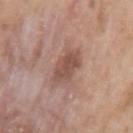{
  "biopsy_status": "not biopsied; imaged during a skin examination",
  "automated_metrics": {
    "area_mm2_approx": 8.0,
    "eccentricity": 0.75,
    "shape_asymmetry": 0.2,
    "border_irregularity_0_10": 2.5,
    "color_variation_0_10": 3.5,
    "peripheral_color_asymmetry": 1.0
  },
  "lesion_size": {
    "long_diameter_mm_approx": 4.0
  },
  "site": "left upper arm",
  "lighting": "white-light",
  "patient": {
    "sex": "male",
    "age_approx": 50
  },
  "image": {
    "source": "total-body photography crop",
    "field_of_view_mm": 15
  }
}